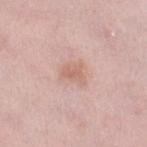Captured during whole-body skin photography for melanoma surveillance; the lesion was not biopsied. The patient is a female in their mid-40s. Cropped from a total-body skin-imaging series; the visible field is about 15 mm. Automated image analysis of the tile measured an area of roughly 4 mm². The analysis additionally found a lesion–skin lightness drop of about 8 and a lesion-to-skin contrast of about 5.5 (normalized; higher = more distinct). The analysis additionally found lesion-presence confidence of about 100/100. Longest diameter approximately 2.5 mm. From the right lower leg. The tile uses white-light illumination.